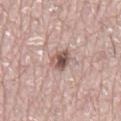Clinical impression: Recorded during total-body skin imaging; not selected for excision or biopsy. Image and clinical context: The lesion is located on the mid back. A male patient, aged approximately 75. This is a white-light tile. The total-body-photography lesion software estimated a lesion color around L≈53 a*≈19 b*≈22 in CIELAB, a lesion–skin lightness drop of about 15, and a normalized border contrast of about 10. The software also gave a border-irregularity index near 2/10, a color-variation rating of about 6.5/10, and peripheral color asymmetry of about 2.5. Cropped from a total-body skin-imaging series; the visible field is about 15 mm.